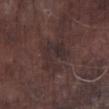{"biopsy_status": "not biopsied; imaged during a skin examination", "lighting": "white-light", "image": {"source": "total-body photography crop", "field_of_view_mm": 15}, "site": "left lower leg", "patient": {"sex": "male", "age_approx": 75}, "lesion_size": {"long_diameter_mm_approx": 4.5}}